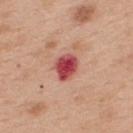Q: Is there a histopathology result?
A: no biopsy performed (imaged during a skin exam)
Q: Automated lesion metrics?
A: a border-irregularity rating of about 2.5/10 and a within-lesion color-variation index near 4.5/10; a detector confidence of about 100 out of 100 that the crop contains a lesion
Q: Patient demographics?
A: female, roughly 40 years of age
Q: Where on the body is the lesion?
A: the upper back
Q: What is the lesion's diameter?
A: ~3.5 mm (longest diameter)
Q: How was this image acquired?
A: 15 mm crop, total-body photography
Q: What lighting was used for the tile?
A: white-light